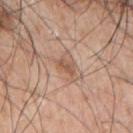Assessment:
Recorded during total-body skin imaging; not selected for excision or biopsy.
Context:
The patient is a male approximately 70 years of age. Captured under white-light illumination. Cropped from a whole-body photographic skin survey; the tile spans about 15 mm. Located on the left arm.